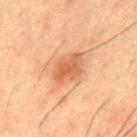workup = total-body-photography surveillance lesion; no biopsy | lesion diameter = about 4 mm | acquisition = ~15 mm crop, total-body skin-cancer survey | subject = male, aged approximately 50 | anatomic site = the mid back | lighting = cross-polarized.